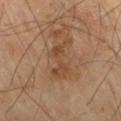The lesion's longest dimension is about 5 mm. A male patient, in their 70s. The lesion is on the right thigh. Automated tile analysis of the lesion measured a lesion area of about 9 mm², an outline eccentricity of about 0.9 (0 = round, 1 = elongated), and two-axis asymmetry of about 0.4. A 15 mm close-up extracted from a 3D total-body photography capture. Imaged with cross-polarized lighting.